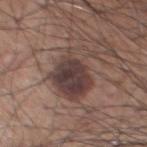{
  "biopsy_status": "not biopsied; imaged during a skin examination",
  "site": "chest",
  "lesion_size": {
    "long_diameter_mm_approx": 6.0
  },
  "lighting": "white-light",
  "image": {
    "source": "total-body photography crop",
    "field_of_view_mm": 15
  },
  "patient": {
    "sex": "male",
    "age_approx": 75
  }
}